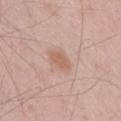Findings:
– follow-up · total-body-photography surveillance lesion; no biopsy
– body site · the leg
– acquisition · ~15 mm crop, total-body skin-cancer survey
– patient · male, aged around 55